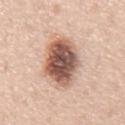Q: Was this lesion biopsied?
A: imaged on a skin check; not biopsied
Q: Lesion location?
A: the chest
Q: What is the imaging modality?
A: ~15 mm crop, total-body skin-cancer survey
Q: What are the patient's age and sex?
A: male, aged 63 to 67